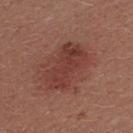Context:
The total-body-photography lesion software estimated a border-irregularity index near 2.5/10, a color-variation rating of about 3.5/10, and a peripheral color-asymmetry measure near 1.5. And it measured lesion-presence confidence of about 100/100. A female patient aged 23–27. The lesion is on the upper back. Approximately 6 mm at its widest. This is a white-light tile. A lesion tile, about 15 mm wide, cut from a 3D total-body photograph.